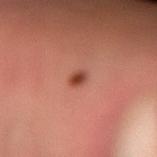Part of a total-body skin-imaging series; this lesion was reviewed on a skin check and was not flagged for biopsy. A roughly 15 mm field-of-view crop from a total-body skin photograph. Captured under cross-polarized illumination. The recorded lesion diameter is about 2 mm. An algorithmic analysis of the crop reported a lesion area of about 2 mm², a shape eccentricity near 0.65, and a symmetry-axis asymmetry near 0.25. The analysis additionally found border irregularity of about 2 on a 0–10 scale, internal color variation of about 2 on a 0–10 scale, and radial color variation of about 0.5. And it measured a classifier nevus-likeness of about 100/100 and lesion-presence confidence of about 100/100. The lesion is on the left forearm. A male subject, in their mid- to late 30s.